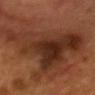Captured during whole-body skin photography for melanoma surveillance; the lesion was not biopsied. A male subject in their mid-50s. The total-body-photography lesion software estimated an average lesion color of about L≈28 a*≈20 b*≈27 (CIELAB), a lesion–skin lightness drop of about 10, and a normalized lesion–skin contrast near 10. The analysis additionally found a color-variation rating of about 6.5/10 and a peripheral color-asymmetry measure near 2. The analysis additionally found a lesion-detection confidence of about 70/100. The lesion is on the head or neck. Cropped from a total-body skin-imaging series; the visible field is about 15 mm.